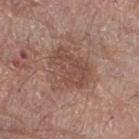The lesion was tiled from a total-body skin photograph and was not biopsied. The patient is a male aged approximately 75. Cropped from a whole-body photographic skin survey; the tile spans about 15 mm. Located on the right thigh.